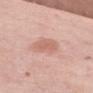Notes:
- notes · imaged on a skin check; not biopsied
- acquisition · ~15 mm crop, total-body skin-cancer survey
- automated lesion analysis · a lesion area of about 7.5 mm², an outline eccentricity of about 0.65 (0 = round, 1 = elongated), and a shape-asymmetry score of about 0.2 (0 = symmetric); a border-irregularity rating of about 2.5/10, a color-variation rating of about 1.5/10, and peripheral color asymmetry of about 0.5; a nevus-likeness score of about 75/100 and a detector confidence of about 100 out of 100 that the crop contains a lesion
- anatomic site · the chest
- lesion size · about 3.5 mm
- patient · female, approximately 65 years of age